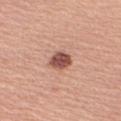| feature | finding |
|---|---|
| follow-up | no biopsy performed (imaged during a skin exam) |
| acquisition | total-body-photography crop, ~15 mm field of view |
| subject | female, approximately 55 years of age |
| location | the right upper arm |